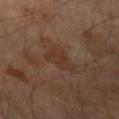{"biopsy_status": "not biopsied; imaged during a skin examination", "site": "arm", "image": {"source": "total-body photography crop", "field_of_view_mm": 15}, "lesion_size": {"long_diameter_mm_approx": 3.5}, "patient": {"sex": "male", "age_approx": 65}}